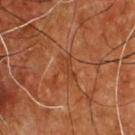<case>
<biopsy_status>not biopsied; imaged during a skin examination</biopsy_status>
<lighting>cross-polarized</lighting>
<image>
  <source>total-body photography crop</source>
  <field_of_view_mm>15</field_of_view_mm>
</image>
<site>chest</site>
<patient>
  <sex>male</sex>
  <age_approx>55</age_approx>
</patient>
<automated_metrics>
  <cielab_L>38</cielab_L>
  <cielab_a>25</cielab_a>
  <cielab_b>34</cielab_b>
  <vs_skin_darker_L>5.0</vs_skin_darker_L>
  <vs_skin_contrast_norm>5.0</vs_skin_contrast_norm>
  <border_irregularity_0_10>4.5</border_irregularity_0_10>
  <peripheral_color_asymmetry>0.0</peripheral_color_asymmetry>
  <nevus_likeness_0_100>0</nevus_likeness_0_100>
  <lesion_detection_confidence_0_100>85</lesion_detection_confidence_0_100>
</automated_metrics>
</case>